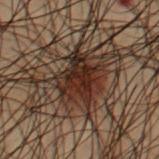Q: Is there a histopathology result?
A: total-body-photography surveillance lesion; no biopsy
Q: What is the anatomic site?
A: the chest
Q: What are the patient's age and sex?
A: male, aged 53–57
Q: How was this image acquired?
A: 15 mm crop, total-body photography